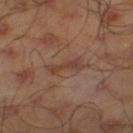Imaged during a routine full-body skin examination; the lesion was not biopsied and no histopathology is available. A male subject in their mid-40s. The lesion-visualizer software estimated an area of roughly 4.5 mm² and an outline eccentricity of about 0.95 (0 = round, 1 = elongated). The software also gave a border-irregularity rating of about 5.5/10, a within-lesion color-variation index near 1.5/10, and radial color variation of about 0. It also reported a classifier nevus-likeness of about 0/100 and lesion-presence confidence of about 95/100. The lesion is on the left thigh. Cropped from a whole-body photographic skin survey; the tile spans about 15 mm.